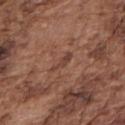A male subject, aged 73–77. A roughly 15 mm field-of-view crop from a total-body skin photograph. The lesion is located on the right upper arm.